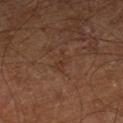Clinical impression:
Captured during whole-body skin photography for melanoma surveillance; the lesion was not biopsied.
Background:
A male subject, approximately 60 years of age. Captured under cross-polarized illumination. From the leg. The lesion-visualizer software estimated border irregularity of about 6.5 on a 0–10 scale, a within-lesion color-variation index near 0/10, and radial color variation of about 0. And it measured lesion-presence confidence of about 90/100. A 15 mm crop from a total-body photograph taken for skin-cancer surveillance.